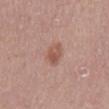notes = total-body-photography surveillance lesion; no biopsy
image source = 15 mm crop, total-body photography
subject = male, roughly 60 years of age
site = the mid back